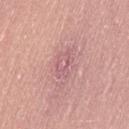Case summary:
- workup: catalogued during a skin exam; not biopsied